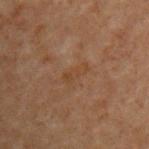Captured during whole-body skin photography for melanoma surveillance; the lesion was not biopsied.